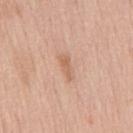The patient is a male in their 60s.
From the chest.
A 15 mm crop from a total-body photograph taken for skin-cancer surveillance.
Longest diameter approximately 3 mm.
Captured under white-light illumination.